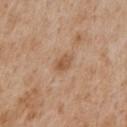Context:
A male patient, in their mid- to late 60s. Located on the chest. Cropped from a whole-body photographic skin survey; the tile spans about 15 mm. The lesion-visualizer software estimated roughly 9 lightness units darker than nearby skin and a normalized lesion–skin contrast near 6.5. About 3 mm across.